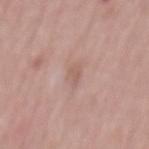The lesion was tiled from a total-body skin photograph and was not biopsied.
The tile uses white-light illumination.
The lesion-visualizer software estimated an eccentricity of roughly 0.85 and a shape-asymmetry score of about 0.35 (0 = symmetric). It also reported a lesion color around L≈58 a*≈19 b*≈24 in CIELAB and a normalized border contrast of about 5. The analysis additionally found a classifier nevus-likeness of about 0/100 and a lesion-detection confidence of about 100/100.
Approximately 3 mm at its widest.
On the mid back.
A 15 mm close-up tile from a total-body photography series done for melanoma screening.
The subject is a male aged 48 to 52.